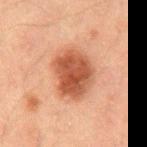A roughly 15 mm field-of-view crop from a total-body skin photograph. The subject is a male aged 48 to 52. An algorithmic analysis of the crop reported a lesion color around L≈41 a*≈23 b*≈29 in CIELAB, a lesion–skin lightness drop of about 12, and a normalized lesion–skin contrast near 10. The software also gave a border-irregularity rating of about 2/10 and a peripheral color-asymmetry measure near 1.5. The analysis additionally found lesion-presence confidence of about 100/100. Located on the mid back. Longest diameter approximately 5 mm. Imaged with cross-polarized lighting.